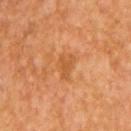The lesion was tiled from a total-body skin photograph and was not biopsied.
The lesion is on the arm.
A male subject about 55 years old.
A close-up tile cropped from a whole-body skin photograph, about 15 mm across.